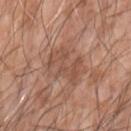No biopsy was performed on this lesion — it was imaged during a full skin examination and was not determined to be concerning. A male subject, in their 60s. A 15 mm close-up tile from a total-body photography series done for melanoma screening. Imaged with white-light lighting. The lesion is located on the left forearm. Approximately 5 mm at its widest.